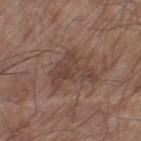Part of a total-body skin-imaging series; this lesion was reviewed on a skin check and was not flagged for biopsy.
The lesion is on the left thigh.
This image is a 15 mm lesion crop taken from a total-body photograph.
Longest diameter approximately 5 mm.
Imaged with white-light lighting.
A male subject approximately 80 years of age.